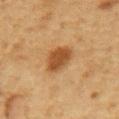Findings:
• notes: no biopsy performed (imaged during a skin exam)
• image source: ~15 mm crop, total-body skin-cancer survey
• subject: female, aged around 45
• size: ~3.5 mm (longest diameter)
• anatomic site: the right forearm
• illumination: cross-polarized